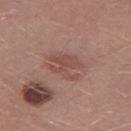Cropped from a whole-body photographic skin survey; the tile spans about 15 mm. A male subject, approximately 30 years of age. The lesion is on the left thigh.